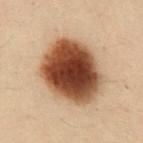Q: Patient demographics?
A: male, roughly 30 years of age
Q: Illumination type?
A: cross-polarized illumination
Q: What is the anatomic site?
A: the abdomen
Q: What is the imaging modality?
A: ~15 mm tile from a whole-body skin photo
Q: What did automated image analysis measure?
A: a lesion area of about 36 mm², an outline eccentricity of about 0.4 (0 = round, 1 = elongated), and two-axis asymmetry of about 0.15; a mean CIELAB color near L≈37 a*≈19 b*≈27 and a normalized border contrast of about 15.5; a border-irregularity rating of about 1.5/10, internal color variation of about 7 on a 0–10 scale, and radial color variation of about 1.5; a nevus-likeness score of about 100/100
Q: How large is the lesion?
A: ~7 mm (longest diameter)